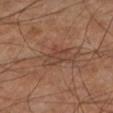workup — total-body-photography surveillance lesion; no biopsy | subject — male, roughly 65 years of age | site — the right lower leg | lesion diameter — ≈4 mm | imaging modality — ~15 mm crop, total-body skin-cancer survey | lighting — cross-polarized illumination.